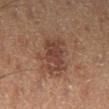No biopsy was performed on this lesion — it was imaged during a full skin examination and was not determined to be concerning. The lesion is located on the right lower leg. A close-up tile cropped from a whole-body skin photograph, about 15 mm across. A male subject aged around 55.